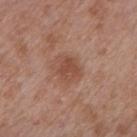Captured during whole-body skin photography for melanoma surveillance; the lesion was not biopsied. Imaged with white-light lighting. A 15 mm crop from a total-body photograph taken for skin-cancer surveillance. Located on the mid back. The patient is a male in their mid-60s. About 3 mm across.